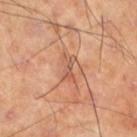Q: Is there a histopathology result?
A: total-body-photography surveillance lesion; no biopsy
Q: Where on the body is the lesion?
A: the left lower leg
Q: What kind of image is this?
A: total-body-photography crop, ~15 mm field of view
Q: Who is the patient?
A: male, aged 68–72
Q: What is the lesion's diameter?
A: about 3.5 mm
Q: What did automated image analysis measure?
A: a lesion color around L≈55 a*≈24 b*≈32 in CIELAB and a normalized border contrast of about 5.5; a within-lesion color-variation index near 6.5/10 and radial color variation of about 2.5; a classifier nevus-likeness of about 0/100 and lesion-presence confidence of about 80/100